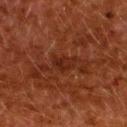| field | value |
|---|---|
| biopsy status | total-body-photography surveillance lesion; no biopsy |
| body site | the upper back |
| imaging modality | ~15 mm tile from a whole-body skin photo |
| lesion size | ≈3 mm |
| subject | female, aged 48–52 |